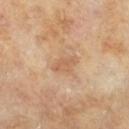Captured during whole-body skin photography for melanoma surveillance; the lesion was not biopsied. Captured under cross-polarized illumination. The lesion's longest dimension is about 3 mm. A male subject aged 63 to 67. On the right lower leg. A lesion tile, about 15 mm wide, cut from a 3D total-body photograph.